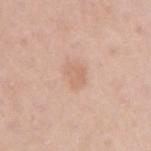Notes:
• location — the arm
• image source — ~15 mm tile from a whole-body skin photo
• subject — female, aged 43 to 47